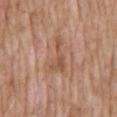Notes:
* follow-up · imaged on a skin check; not biopsied
* imaging modality · ~15 mm tile from a whole-body skin photo
* subject · male, roughly 60 years of age
* diameter · about 4.5 mm
* illumination · white-light illumination
* anatomic site · the abdomen